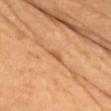Q: Was this lesion biopsied?
A: total-body-photography surveillance lesion; no biopsy
Q: Illumination type?
A: cross-polarized illumination
Q: What are the patient's age and sex?
A: female, aged around 50
Q: Lesion size?
A: about 2.5 mm
Q: How was this image acquired?
A: total-body-photography crop, ~15 mm field of view
Q: Lesion location?
A: the chest